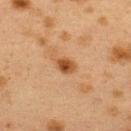| key | value |
|---|---|
| diameter | about 2.5 mm |
| subject | female, aged approximately 40 |
| illumination | cross-polarized |
| automated metrics | a shape eccentricity near 0.75; an average lesion color of about L≈41 a*≈21 b*≈33 (CIELAB) and a lesion-to-skin contrast of about 9.5 (normalized; higher = more distinct); a color-variation rating of about 3.5/10 and peripheral color asymmetry of about 1 |
| anatomic site | the back |
| acquisition | 15 mm crop, total-body photography |